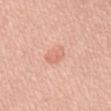image source: 15 mm crop, total-body photography; site: the mid back; subject: male, roughly 50 years of age.